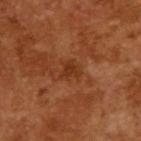Q: Is there a histopathology result?
A: no biopsy performed (imaged during a skin exam)
Q: How was the tile lit?
A: cross-polarized illumination
Q: What kind of image is this?
A: 15 mm crop, total-body photography
Q: What are the patient's age and sex?
A: male, approximately 65 years of age